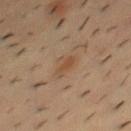| feature | finding |
|---|---|
| notes | catalogued during a skin exam; not biopsied |
| site | the upper back |
| imaging modality | ~15 mm crop, total-body skin-cancer survey |
| image-analysis metrics | an outline eccentricity of about 0.65 (0 = round, 1 = elongated) and a shape-asymmetry score of about 0.3 (0 = symmetric); a mean CIELAB color near L≈41 a*≈16 b*≈28, roughly 6 lightness units darker than nearby skin, and a normalized lesion–skin contrast near 6; an automated nevus-likeness rating near 60 out of 100 |
| patient | male, in their mid- to late 50s |
| diameter | about 2.5 mm |